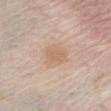The lesion was tiled from a total-body skin photograph and was not biopsied.
A close-up tile cropped from a whole-body skin photograph, about 15 mm across.
On the chest.
The tile uses white-light illumination.
The lesion's longest dimension is about 3 mm.
A female patient aged 63–67.